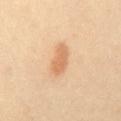Assessment:
Part of a total-body skin-imaging series; this lesion was reviewed on a skin check and was not flagged for biopsy.
Background:
A female subject roughly 40 years of age. The lesion is on the back. This is a cross-polarized tile. A lesion tile, about 15 mm wide, cut from a 3D total-body photograph. The lesion's longest dimension is about 4 mm. An algorithmic analysis of the crop reported a footprint of about 6 mm² and an eccentricity of roughly 0.9. And it measured an automated nevus-likeness rating near 90 out of 100 and lesion-presence confidence of about 100/100.